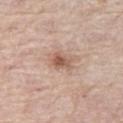The lesion was tiled from a total-body skin photograph and was not biopsied. This image is a 15 mm lesion crop taken from a total-body photograph. Automated image analysis of the tile measured an outline eccentricity of about 0.65 (0 = round, 1 = elongated) and two-axis asymmetry of about 0.3. It also reported border irregularity of about 3 on a 0–10 scale, internal color variation of about 4.5 on a 0–10 scale, and radial color variation of about 1.5. Located on the left thigh. This is a white-light tile. A female patient approximately 70 years of age.